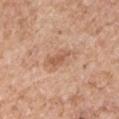lesion size=about 4.5 mm; lighting=white-light; image=~15 mm crop, total-body skin-cancer survey; anatomic site=the right upper arm; patient=male, aged 53 to 57.